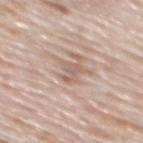{
  "biopsy_status": "not biopsied; imaged during a skin examination",
  "image": {
    "source": "total-body photography crop",
    "field_of_view_mm": 15
  },
  "patient": {
    "sex": "male",
    "age_approx": 80
  },
  "automated_metrics": {
    "area_mm2_approx": 4.5,
    "eccentricity": 0.7,
    "shape_asymmetry": 0.4,
    "cielab_L": 61,
    "cielab_a": 16,
    "cielab_b": 24,
    "vs_skin_darker_L": 8.0,
    "border_irregularity_0_10": 5.0,
    "color_variation_0_10": 1.5,
    "peripheral_color_asymmetry": 0.5,
    "nevus_likeness_0_100": 0,
    "lesion_detection_confidence_0_100": 55
  },
  "site": "mid back",
  "lesion_size": {
    "long_diameter_mm_approx": 3.0
  }
}